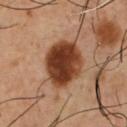Impression:
Recorded during total-body skin imaging; not selected for excision or biopsy.